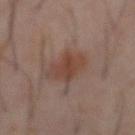Notes:
- workup · imaged on a skin check; not biopsied
- size · ≈4 mm
- subject · male, in their 60s
- anatomic site · the abdomen
- image-analysis metrics · a footprint of about 10 mm², an eccentricity of roughly 0.6, and two-axis asymmetry of about 0.3
- acquisition · total-body-photography crop, ~15 mm field of view